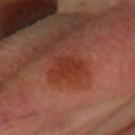notes: imaged on a skin check; not biopsied
diameter: ≈3.5 mm
image source: 15 mm crop, total-body photography
image-analysis metrics: a lesion area of about 6.5 mm², a shape eccentricity near 0.65, and a symmetry-axis asymmetry near 0.3; a border-irregularity index near 3.5/10 and a peripheral color-asymmetry measure near 0.5
illumination: cross-polarized illumination
patient: female, aged approximately 60
location: the head or neck